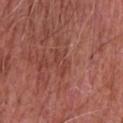The lesion was tiled from a total-body skin photograph and was not biopsied.
Automated image analysis of the tile measured an eccentricity of roughly 0.85 and two-axis asymmetry of about 0.4. And it measured a nevus-likeness score of about 0/100 and lesion-presence confidence of about 80/100.
A male subject in their mid- to late 60s.
On the chest.
This is a white-light tile.
Longest diameter approximately 3.5 mm.
A roughly 15 mm field-of-view crop from a total-body skin photograph.